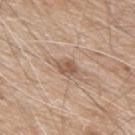Captured during whole-body skin photography for melanoma surveillance; the lesion was not biopsied.
The lesion is on the upper back.
A 15 mm close-up tile from a total-body photography series done for melanoma screening.
A male patient approximately 80 years of age.